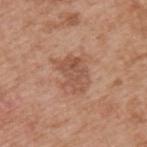  biopsy_status: not biopsied; imaged during a skin examination
  patient:
    sex: male
    age_approx: 55
  lighting: white-light
  site: back
  automated_metrics:
    vs_skin_darker_L: 9.0
    lesion_detection_confidence_0_100: 100
  image:
    source: total-body photography crop
    field_of_view_mm: 15